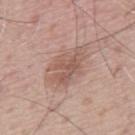biopsy status = imaged on a skin check; not biopsied
tile lighting = white-light illumination
anatomic site = the upper back
imaging modality = ~15 mm tile from a whole-body skin photo
lesion diameter = ≈5 mm
subject = male, aged 63 to 67